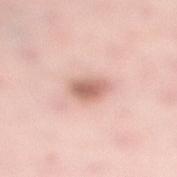The lesion was tiled from a total-body skin photograph and was not biopsied. Cropped from a total-body skin-imaging series; the visible field is about 15 mm. On the leg. Automated tile analysis of the lesion measured a footprint of about 6 mm². It also reported an automated nevus-likeness rating near 85 out of 100 and lesion-presence confidence of about 100/100. A female patient, aged around 30. Measured at roughly 3 mm in maximum diameter. Imaged with white-light lighting.